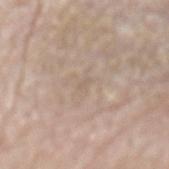patient = male, aged approximately 75; lesion diameter = ≈1.5 mm; body site = the mid back; automated lesion analysis = an average lesion color of about L≈60 a*≈13 b*≈25 (CIELAB), roughly 4 lightness units darker than nearby skin, and a normalized lesion–skin contrast near 3; image = ~15 mm tile from a whole-body skin photo.